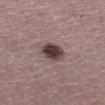follow-up — total-body-photography surveillance lesion; no biopsy
acquisition — 15 mm crop, total-body photography
subject — male, roughly 60 years of age
size — ~3.5 mm (longest diameter)
automated metrics — a footprint of about 8.5 mm² and an outline eccentricity of about 0.6 (0 = round, 1 = elongated); a border-irregularity rating of about 1.5/10, internal color variation of about 6.5 on a 0–10 scale, and a peripheral color-asymmetry measure near 2; a nevus-likeness score of about 60/100 and a detector confidence of about 100 out of 100 that the crop contains a lesion
tile lighting — white-light illumination
location — the abdomen